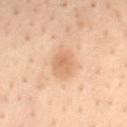{"biopsy_status": "not biopsied; imaged during a skin examination", "site": "mid back", "lesion_size": {"long_diameter_mm_approx": 3.0}, "image": {"source": "total-body photography crop", "field_of_view_mm": 15}, "patient": {"sex": "male", "age_approx": 40}, "lighting": "cross-polarized", "automated_metrics": {"area_mm2_approx": 6.5, "eccentricity": 0.45, "shape_asymmetry": 0.2, "border_irregularity_0_10": 1.5, "peripheral_color_asymmetry": 1.0, "lesion_detection_confidence_0_100": 100}}